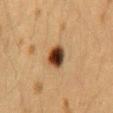No biopsy was performed on this lesion — it was imaged during a full skin examination and was not determined to be concerning. Imaged with cross-polarized lighting. A 15 mm close-up extracted from a 3D total-body photography capture. From the back. Approximately 3 mm at its widest. A female patient approximately 40 years of age.